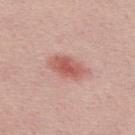Part of a total-body skin-imaging series; this lesion was reviewed on a skin check and was not flagged for biopsy. Cropped from a whole-body photographic skin survey; the tile spans about 15 mm. Imaged with white-light lighting. The lesion's longest dimension is about 4.5 mm. Automated image analysis of the tile measured a nevus-likeness score of about 60/100 and lesion-presence confidence of about 100/100. A male subject roughly 30 years of age.